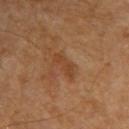Case summary:
* follow-up: catalogued during a skin exam; not biopsied
* illumination: cross-polarized illumination
* location: the upper back
* patient: female, aged approximately 65
* image source: 15 mm crop, total-body photography
* lesion size: ~3.5 mm (longest diameter)